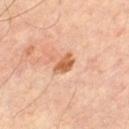  biopsy_status: not biopsied; imaged during a skin examination
  lighting: cross-polarized
  automated_metrics:
    area_mm2_approx: 3.5
    eccentricity: 0.75
    shape_asymmetry: 0.35
    vs_skin_darker_L: 13.0
    vs_skin_contrast_norm: 9.0
  image:
    source: total-body photography crop
    field_of_view_mm: 15
  lesion_size:
    long_diameter_mm_approx: 2.5
  site: leg
  patient:
    sex: male
    age_approx: 65